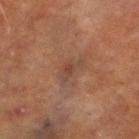Q: What is the lesion's diameter?
A: about 3 mm
Q: How was this image acquired?
A: ~15 mm crop, total-body skin-cancer survey
Q: What is the anatomic site?
A: the leg
Q: Patient demographics?
A: male, in their 70s